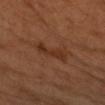Impression:
No biopsy was performed on this lesion — it was imaged during a full skin examination and was not determined to be concerning.
Image and clinical context:
The total-body-photography lesion software estimated an area of roughly 7 mm² and an eccentricity of roughly 0.95. And it measured a mean CIELAB color near L≈33 a*≈22 b*≈31, a lesion–skin lightness drop of about 7, and a normalized border contrast of about 6.5. From the left forearm. Imaged with cross-polarized lighting. A 15 mm close-up extracted from a 3D total-body photography capture. A female subject aged approximately 60.